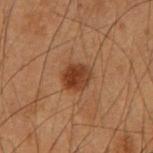Clinical impression:
No biopsy was performed on this lesion — it was imaged during a full skin examination and was not determined to be concerning.
Background:
A close-up tile cropped from a whole-body skin photograph, about 15 mm across. A male subject aged approximately 55. Located on the right forearm. This is a cross-polarized tile.